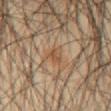notes=catalogued during a skin exam; not biopsied
body site=the abdomen
imaging modality=total-body-photography crop, ~15 mm field of view
subject=male, in their 60s